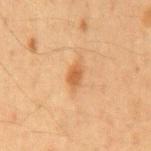Assessment:
Part of a total-body skin-imaging series; this lesion was reviewed on a skin check and was not flagged for biopsy.
Image and clinical context:
Captured under cross-polarized illumination. Located on the back. A male patient approximately 65 years of age. This image is a 15 mm lesion crop taken from a total-body photograph. About 3.5 mm across.